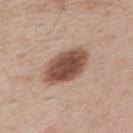Assessment: Imaged during a routine full-body skin examination; the lesion was not biopsied and no histopathology is available. Context: This is a white-light tile. The patient is a male approximately 55 years of age. The lesion is located on the left upper arm. A lesion tile, about 15 mm wide, cut from a 3D total-body photograph. An algorithmic analysis of the crop reported an area of roughly 17 mm² and an eccentricity of roughly 0.75. And it measured a mean CIELAB color near L≈50 a*≈20 b*≈27, about 17 CIELAB-L* units darker than the surrounding skin, and a lesion-to-skin contrast of about 11 (normalized; higher = more distinct). The analysis additionally found border irregularity of about 1.5 on a 0–10 scale and internal color variation of about 5 on a 0–10 scale. And it measured a classifier nevus-likeness of about 95/100.